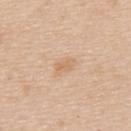Case summary:
– notes · no biopsy performed (imaged during a skin exam)
– acquisition · 15 mm crop, total-body photography
– subject · female, aged approximately 45
– lesion diameter · ≈3 mm
– location · the upper back
– illumination · white-light illumination